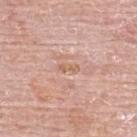biopsy status: no biopsy performed (imaged during a skin exam)